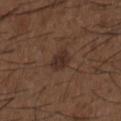notes: no biopsy performed (imaged during a skin exam)